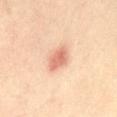{
  "biopsy_status": "not biopsied; imaged during a skin examination",
  "image": {
    "source": "total-body photography crop",
    "field_of_view_mm": 15
  },
  "lesion_size": {
    "long_diameter_mm_approx": 3.0
  },
  "lighting": "cross-polarized",
  "site": "abdomen",
  "patient": {
    "sex": "female",
    "age_approx": 40
  },
  "automated_metrics": {
    "area_mm2_approx": 5.5,
    "eccentricity": 0.75,
    "shape_asymmetry": 0.2,
    "border_irregularity_0_10": 2.0,
    "color_variation_0_10": 2.5,
    "peripheral_color_asymmetry": 1.0,
    "nevus_likeness_0_100": 55,
    "lesion_detection_confidence_0_100": 100
  }
}